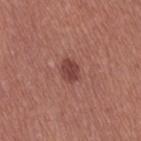Recorded during total-body skin imaging; not selected for excision or biopsy.
An algorithmic analysis of the crop reported a lesion area of about 4.5 mm², an eccentricity of roughly 0.65, and two-axis asymmetry of about 0.2. The software also gave a border-irregularity index near 2/10 and a color-variation rating of about 2/10.
The subject is a female aged 48 to 52.
The lesion is located on the right thigh.
A close-up tile cropped from a whole-body skin photograph, about 15 mm across.
The tile uses white-light illumination.
The lesion's longest dimension is about 2.5 mm.